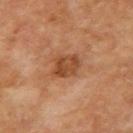| field | value |
|---|---|
| follow-up | catalogued during a skin exam; not biopsied |
| image | 15 mm crop, total-body photography |
| patient | male, roughly 70 years of age |
| illumination | cross-polarized illumination |
| site | the arm |
| lesion size | ≈3.5 mm |